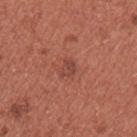{"biopsy_status": "not biopsied; imaged during a skin examination", "patient": {"sex": "female", "age_approx": 35}, "site": "right upper arm", "image": {"source": "total-body photography crop", "field_of_view_mm": 15}, "lesion_size": {"long_diameter_mm_approx": 2.5}, "automated_metrics": {"border_irregularity_0_10": 3.0, "color_variation_0_10": 3.0, "peripheral_color_asymmetry": 1.0}}